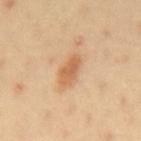This lesion was catalogued during total-body skin photography and was not selected for biopsy. On the back. Captured under cross-polarized illumination. A lesion tile, about 15 mm wide, cut from a 3D total-body photograph. Approximately 4 mm at its widest. A male subject approximately 40 years of age.